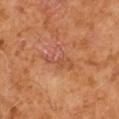A male patient about 60 years old.
The total-body-photography lesion software estimated a lesion color around L≈50 a*≈25 b*≈32 in CIELAB, roughly 6 lightness units darker than nearby skin, and a normalized border contrast of about 5. The analysis additionally found a border-irregularity rating of about 5/10 and a within-lesion color-variation index near 4.5/10. It also reported a detector confidence of about 100 out of 100 that the crop contains a lesion.
A 15 mm close-up tile from a total-body photography series done for melanoma screening.
This is a cross-polarized tile.
The lesion is on the right arm.